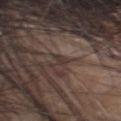biopsy status = imaged on a skin check; not biopsied | size = ≈1.5 mm | site = the head or neck | acquisition = ~15 mm tile from a whole-body skin photo | subject = male, in their mid- to late 50s | illumination = white-light illumination.